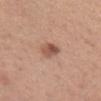image-analysis metrics: a lesion area of about 4.5 mm² and an outline eccentricity of about 0.85 (0 = round, 1 = elongated)
lighting: white-light illumination
imaging modality: ~15 mm crop, total-body skin-cancer survey
subject: female, aged 28 to 32
location: the arm
diameter: ~3.5 mm (longest diameter)